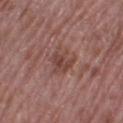The subject is a female aged around 70. A roughly 15 mm field-of-view crop from a total-body skin photograph. The lesion is located on the left thigh.